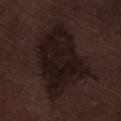This lesion was catalogued during total-body skin photography and was not selected for biopsy.
A 15 mm close-up tile from a total-body photography series done for melanoma screening.
From the left lower leg.
An algorithmic analysis of the crop reported an area of roughly 38 mm² and a symmetry-axis asymmetry near 0.3. The software also gave an average lesion color of about L≈14 a*≈12 b*≈12 (CIELAB), a lesion–skin lightness drop of about 7, and a lesion-to-skin contrast of about 11.5 (normalized; higher = more distinct).
The lesion's longest dimension is about 8.5 mm.
Captured under white-light illumination.
The patient is a male aged 68–72.